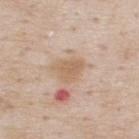The lesion was photographed on a routine skin check and not biopsied; there is no pathology result.
Located on the upper back.
The patient is a male about 80 years old.
This is a white-light tile.
A close-up tile cropped from a whole-body skin photograph, about 15 mm across.